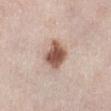Assessment: Captured during whole-body skin photography for melanoma surveillance; the lesion was not biopsied. Acquisition and patient details: From the left lower leg. Cropped from a total-body skin-imaging series; the visible field is about 15 mm. Approximately 3.5 mm at its widest. A female patient aged 63 to 67.